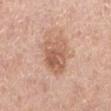- notes: imaged on a skin check; not biopsied
- patient: female, aged approximately 65
- size: about 4.5 mm
- image-analysis metrics: roughly 10 lightness units darker than nearby skin; border irregularity of about 2 on a 0–10 scale and peripheral color asymmetry of about 2
- body site: the left lower leg
- imaging modality: ~15 mm crop, total-body skin-cancer survey